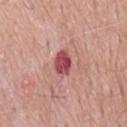Q: Was a biopsy performed?
A: imaged on a skin check; not biopsied
Q: How was this image acquired?
A: ~15 mm crop, total-body skin-cancer survey
Q: Automated lesion metrics?
A: an area of roughly 6 mm², an outline eccentricity of about 0.75 (0 = round, 1 = elongated), and a symmetry-axis asymmetry near 0.25; internal color variation of about 4 on a 0–10 scale and peripheral color asymmetry of about 1.5
Q: Illumination type?
A: white-light illumination
Q: Where on the body is the lesion?
A: the mid back
Q: Patient demographics?
A: male, approximately 65 years of age